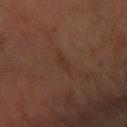A male subject, aged approximately 60.
A roughly 15 mm field-of-view crop from a total-body skin photograph.
This is a cross-polarized tile.
On the right forearm.